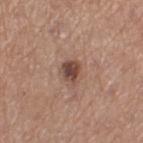- notes — imaged on a skin check; not biopsied
- lighting — white-light
- imaging modality — ~15 mm crop, total-body skin-cancer survey
- anatomic site — the left thigh
- subject — female, aged 53 to 57
- automated metrics — a lesion area of about 5 mm² and a symmetry-axis asymmetry near 0.15; border irregularity of about 1.5 on a 0–10 scale, a within-lesion color-variation index near 5.5/10, and peripheral color asymmetry of about 2
- diameter — ≈3 mm